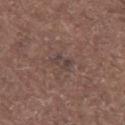{
  "patient": {
    "sex": "male",
    "age_approx": 80
  },
  "lighting": "white-light",
  "image": {
    "source": "total-body photography crop",
    "field_of_view_mm": 15
  },
  "automated_metrics": {
    "cielab_L": 41,
    "cielab_a": 14,
    "cielab_b": 18,
    "vs_skin_darker_L": 6.0,
    "vs_skin_contrast_norm": 6.0,
    "border_irregularity_0_10": 3.0,
    "color_variation_0_10": 3.5,
    "nevus_likeness_0_100": 0,
    "lesion_detection_confidence_0_100": 85
  },
  "lesion_size": {
    "long_diameter_mm_approx": 2.5
  },
  "site": "chest"
}